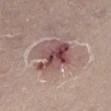– workup · total-body-photography surveillance lesion; no biopsy
– lesion size · ~4.5 mm (longest diameter)
– location · the left lower leg
– image · ~15 mm tile from a whole-body skin photo
– subject · male, aged 63 to 67